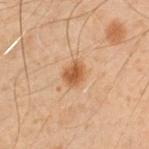Case summary:
- notes — no biopsy performed (imaged during a skin exam)
- body site — the right upper arm
- imaging modality — ~15 mm crop, total-body skin-cancer survey
- patient — male, aged 48–52